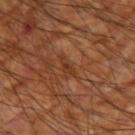Findings:
- follow-up · no biopsy performed (imaged during a skin exam)
- subject · male, aged 58–62
- location · the right upper arm
- image source · total-body-photography crop, ~15 mm field of view
- tile lighting · cross-polarized
- automated lesion analysis · a nevus-likeness score of about 0/100 and a lesion-detection confidence of about 55/100